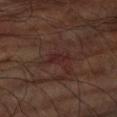Clinical impression: Recorded during total-body skin imaging; not selected for excision or biopsy. Clinical summary: A region of skin cropped from a whole-body photographic capture, roughly 15 mm wide. Measured at roughly 3 mm in maximum diameter. On the left upper arm. The lesion-visualizer software estimated a footprint of about 3.5 mm², an outline eccentricity of about 0.85 (0 = round, 1 = elongated), and a symmetry-axis asymmetry near 0.3. The software also gave border irregularity of about 3.5 on a 0–10 scale, a within-lesion color-variation index near 1/10, and peripheral color asymmetry of about 0. A male patient roughly 60 years of age.